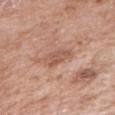Part of a total-body skin-imaging series; this lesion was reviewed on a skin check and was not flagged for biopsy.
Captured under white-light illumination.
From the right upper arm.
The patient is a female aged 68–72.
Measured at roughly 3.5 mm in maximum diameter.
This image is a 15 mm lesion crop taken from a total-body photograph.